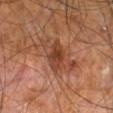The lesion is on the leg. The patient is a male aged around 60. This image is a 15 mm lesion crop taken from a total-body photograph.